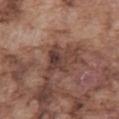{"site": "abdomen", "image": {"source": "total-body photography crop", "field_of_view_mm": 15}, "patient": {"sex": "male", "age_approx": 75}, "lighting": "white-light"}